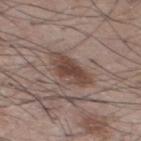<case>
  <biopsy_status>not biopsied; imaged during a skin examination</biopsy_status>
  <lesion_size>
    <long_diameter_mm_approx>5.0</long_diameter_mm_approx>
  </lesion_size>
  <automated_metrics>
    <area_mm2_approx>11.0</area_mm2_approx>
    <eccentricity>0.8</eccentricity>
    <shape_asymmetry>0.25</shape_asymmetry>
    <cielab_L>45</cielab_L>
    <cielab_a>16</cielab_a>
    <cielab_b>23</cielab_b>
    <vs_skin_darker_L>10.0</vs_skin_darker_L>
    <vs_skin_contrast_norm>8.5</vs_skin_contrast_norm>
    <border_irregularity_0_10>3.0</border_irregularity_0_10>
    <color_variation_0_10>4.0</color_variation_0_10>
    <peripheral_color_asymmetry>1.5</peripheral_color_asymmetry>
    <nevus_likeness_0_100>100</nevus_likeness_0_100>
    <lesion_detection_confidence_0_100>100</lesion_detection_confidence_0_100>
  </automated_metrics>
  <site>chest</site>
  <image>
    <source>total-body photography crop</source>
    <field_of_view_mm>15</field_of_view_mm>
  </image>
  <patient>
    <sex>male</sex>
    <age_approx>55</age_approx>
  </patient>
</case>